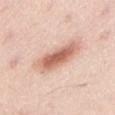notes: no biopsy performed (imaged during a skin exam)
tile lighting: white-light
lesion size: about 7 mm
site: the abdomen
subject: female, roughly 50 years of age
acquisition: ~15 mm crop, total-body skin-cancer survey